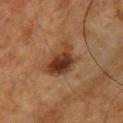Q: Was a biopsy performed?
A: imaged on a skin check; not biopsied
Q: How was this image acquired?
A: ~15 mm crop, total-body skin-cancer survey
Q: How large is the lesion?
A: ≈4.5 mm
Q: Who is the patient?
A: male, aged approximately 60
Q: Automated lesion metrics?
A: a lesion area of about 10 mm², an eccentricity of roughly 0.75, and a shape-asymmetry score of about 0.4 (0 = symmetric); a lesion–skin lightness drop of about 11 and a lesion-to-skin contrast of about 10.5 (normalized; higher = more distinct); a nevus-likeness score of about 95/100 and lesion-presence confidence of about 100/100
Q: What is the anatomic site?
A: the front of the torso
Q: Illumination type?
A: cross-polarized illumination